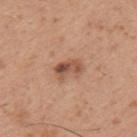* follow-up: no biopsy performed (imaged during a skin exam)
* tile lighting: white-light
* patient: male, about 30 years old
* body site: the arm
* size: ~3.5 mm (longest diameter)
* image source: ~15 mm tile from a whole-body skin photo